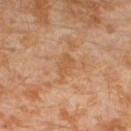{"biopsy_status": "not biopsied; imaged during a skin examination", "automated_metrics": {"area_mm2_approx": 4.0, "eccentricity": 0.8, "shape_asymmetry": 0.3, "border_irregularity_0_10": 3.0, "color_variation_0_10": 1.5, "peripheral_color_asymmetry": 0.5}, "patient": {"sex": "male", "age_approx": 30}, "image": {"source": "total-body photography crop", "field_of_view_mm": 15}, "lighting": "cross-polarized", "site": "left lower leg"}